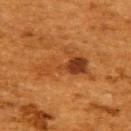Captured during whole-body skin photography for melanoma surveillance; the lesion was not biopsied. A female subject, about 50 years old. From the upper back. A region of skin cropped from a whole-body photographic capture, roughly 15 mm wide.